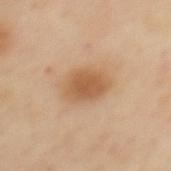No biopsy was performed on this lesion — it was imaged during a full skin examination and was not determined to be concerning. Captured under cross-polarized illumination. The lesion's longest dimension is about 4.5 mm. A roughly 15 mm field-of-view crop from a total-body skin photograph. From the upper back. A female patient approximately 40 years of age.